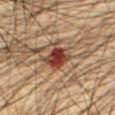follow-up: catalogued during a skin exam; not biopsied
acquisition: ~15 mm crop, total-body skin-cancer survey
anatomic site: the front of the torso
patient: male, aged 63 to 67
image-analysis metrics: an average lesion color of about L≈33 a*≈22 b*≈25 (CIELAB) and a lesion-to-skin contrast of about 12.5 (normalized; higher = more distinct); peripheral color asymmetry of about 1.5
lesion size: about 3.5 mm